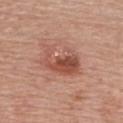{
  "biopsy_status": "not biopsied; imaged during a skin examination",
  "automated_metrics": {
    "area_mm2_approx": 14.0,
    "eccentricity": 0.75,
    "shape_asymmetry": 0.35,
    "border_irregularity_0_10": 5.0,
    "peripheral_color_asymmetry": 2.0
  },
  "site": "front of the torso",
  "lesion_size": {
    "long_diameter_mm_approx": 5.0
  },
  "lighting": "white-light",
  "image": {
    "source": "total-body photography crop",
    "field_of_view_mm": 15
  },
  "patient": {
    "sex": "female",
    "age_approx": 65
  }
}